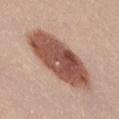{
  "biopsy_status": "not biopsied; imaged during a skin examination",
  "lesion_size": {
    "long_diameter_mm_approx": 9.5
  },
  "site": "right thigh",
  "patient": {
    "sex": "female",
    "age_approx": 25
  },
  "image": {
    "source": "total-body photography crop",
    "field_of_view_mm": 15
  },
  "lighting": "white-light"
}